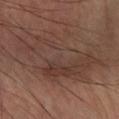follow-up: catalogued during a skin exam; not biopsied | body site: the left forearm | size: ~11 mm (longest diameter) | TBP lesion metrics: roughly 6 lightness units darker than nearby skin and a lesion-to-skin contrast of about 5.5 (normalized; higher = more distinct) | imaging modality: total-body-photography crop, ~15 mm field of view | patient: male, aged around 60.